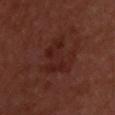Q: Was a biopsy performed?
A: imaged on a skin check; not biopsied
Q: What is the imaging modality?
A: total-body-photography crop, ~15 mm field of view
Q: What is the anatomic site?
A: the upper back
Q: Patient demographics?
A: male, aged 48–52